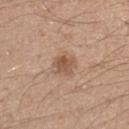The lesion is located on the left forearm. Automated image analysis of the tile measured a lesion color around L≈54 a*≈19 b*≈30 in CIELAB, roughly 10 lightness units darker than nearby skin, and a lesion-to-skin contrast of about 7 (normalized; higher = more distinct). The analysis additionally found a border-irregularity index near 2/10, a color-variation rating of about 3.5/10, and peripheral color asymmetry of about 1. A male subject in their mid-40s. The tile uses white-light illumination. This image is a 15 mm lesion crop taken from a total-body photograph. The recorded lesion diameter is about 3 mm.